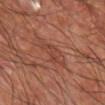Imaged during a routine full-body skin examination; the lesion was not biopsied and no histopathology is available.
The lesion-visualizer software estimated a lesion area of about 7 mm², an eccentricity of roughly 0.8, and a shape-asymmetry score of about 0.35 (0 = symmetric). The analysis additionally found a lesion color around L≈43 a*≈25 b*≈29 in CIELAB and a lesion–skin lightness drop of about 6.
The patient is a male aged around 65.
About 4 mm across.
From the left forearm.
The tile uses cross-polarized illumination.
A 15 mm close-up extracted from a 3D total-body photography capture.